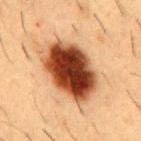Q: Was a biopsy performed?
A: no biopsy performed (imaged during a skin exam)
Q: What is the lesion's diameter?
A: about 8 mm
Q: Automated lesion metrics?
A: a shape eccentricity near 0.8 and two-axis asymmetry of about 0.1; a border-irregularity rating of about 1.5/10 and a color-variation rating of about 10/10; a nevus-likeness score of about 100/100 and a lesion-detection confidence of about 100/100
Q: What lighting was used for the tile?
A: cross-polarized
Q: Who is the patient?
A: male, aged around 55
Q: What is the imaging modality?
A: ~15 mm tile from a whole-body skin photo
Q: Where on the body is the lesion?
A: the chest